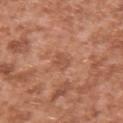Part of a total-body skin-imaging series; this lesion was reviewed on a skin check and was not flagged for biopsy. Automated image analysis of the tile measured a footprint of about 3.5 mm², an outline eccentricity of about 0.8 (0 = round, 1 = elongated), and a symmetry-axis asymmetry near 0.35. It also reported border irregularity of about 3.5 on a 0–10 scale, a within-lesion color-variation index near 1/10, and radial color variation of about 0. Cropped from a total-body skin-imaging series; the visible field is about 15 mm. From the right upper arm. The patient is a male in their mid-40s.